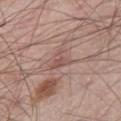biopsy_status: not biopsied; imaged during a skin examination
site: right thigh
lesion_size:
  long_diameter_mm_approx: 3.0
lighting: white-light
image:
  source: total-body photography crop
  field_of_view_mm: 15
automated_metrics:
  area_mm2_approx: 4.0
  eccentricity: 0.5
  shape_asymmetry: 0.55
  cielab_L: 52
  cielab_a: 20
  cielab_b: 22
  vs_skin_contrast_norm: 5.5
  color_variation_0_10: 2.5
  peripheral_color_asymmetry: 1.0
patient:
  sex: male
  age_approx: 65